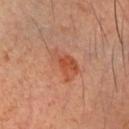Q: Was a biopsy performed?
A: total-body-photography surveillance lesion; no biopsy
Q: Automated lesion metrics?
A: about 7 CIELAB-L* units darker than the surrounding skin and a normalized border contrast of about 6; border irregularity of about 3 on a 0–10 scale, a color-variation rating of about 5.5/10, and peripheral color asymmetry of about 1.5
Q: Who is the patient?
A: male, about 70 years old
Q: What lighting was used for the tile?
A: cross-polarized
Q: Where on the body is the lesion?
A: the head or neck
Q: How was this image acquired?
A: total-body-photography crop, ~15 mm field of view